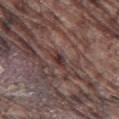Case summary:
- biopsy status — total-body-photography surveillance lesion; no biopsy
- TBP lesion metrics — an area of roughly 2.5 mm² and a shape-asymmetry score of about 0.3 (0 = symmetric); about 9 CIELAB-L* units darker than the surrounding skin
- image — ~15 mm tile from a whole-body skin photo
- diameter — about 2.5 mm
- patient — male, approximately 75 years of age
- location — the leg